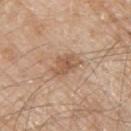Impression:
Recorded during total-body skin imaging; not selected for excision or biopsy.
Clinical summary:
Located on the right upper arm. An algorithmic analysis of the crop reported an area of roughly 6.5 mm² and a shape-asymmetry score of about 0.3 (0 = symmetric). It also reported about 10 CIELAB-L* units darker than the surrounding skin. The software also gave radial color variation of about 1. It also reported an automated nevus-likeness rating near 20 out of 100 and lesion-presence confidence of about 100/100. The subject is a male aged around 80. Approximately 3.5 mm at its widest. Cropped from a total-body skin-imaging series; the visible field is about 15 mm.